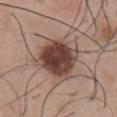Recorded during total-body skin imaging; not selected for excision or biopsy.
Measured at roughly 5.5 mm in maximum diameter.
This is a white-light tile.
On the chest.
A male subject aged around 30.
Automated image analysis of the tile measured an area of roughly 21 mm² and a shape-asymmetry score of about 0.15 (0 = symmetric). It also reported a normalized border contrast of about 12.
A lesion tile, about 15 mm wide, cut from a 3D total-body photograph.